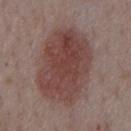The recorded lesion diameter is about 8.5 mm. The lesion is on the chest. The patient is a male aged approximately 75. Cropped from a whole-body photographic skin survey; the tile spans about 15 mm.